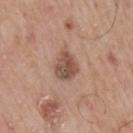Context:
About 3.5 mm across. The total-body-photography lesion software estimated border irregularity of about 2 on a 0–10 scale and a within-lesion color-variation index near 6.5/10. Cropped from a total-body skin-imaging series; the visible field is about 15 mm. A male patient, aged approximately 75. The lesion is on the mid back. Captured under white-light illumination.